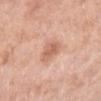– notes: imaged on a skin check; not biopsied
– lesion size: ~2.5 mm (longest diameter)
– illumination: white-light
– subject: female, aged 53–57
– acquisition: ~15 mm crop, total-body skin-cancer survey
– location: the right lower leg
– image-analysis metrics: an area of roughly 5.5 mm²; a border-irregularity rating of about 2.5/10 and a color-variation rating of about 3/10; a classifier nevus-likeness of about 55/100 and a lesion-detection confidence of about 100/100